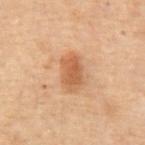Part of a total-body skin-imaging series; this lesion was reviewed on a skin check and was not flagged for biopsy.